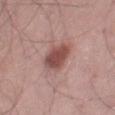Recorded during total-body skin imaging; not selected for excision or biopsy.
The lesion's longest dimension is about 4 mm.
The lesion is on the right thigh.
The tile uses white-light illumination.
A region of skin cropped from a whole-body photographic capture, roughly 15 mm wide.
The subject is a male aged around 70.